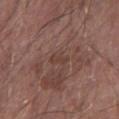notes — imaged on a skin check; not biopsied
site — the right forearm
patient — male, aged 63 to 67
acquisition — 15 mm crop, total-body photography
TBP lesion metrics — an average lesion color of about L≈40 a*≈18 b*≈23 (CIELAB), roughly 6 lightness units darker than nearby skin, and a normalized lesion–skin contrast near 5; a color-variation rating of about 0/10 and radial color variation of about 0; an automated nevus-likeness rating near 0 out of 100 and a lesion-detection confidence of about 65/100
tile lighting — white-light
lesion diameter — about 2.5 mm